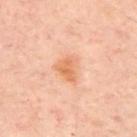Longest diameter approximately 3 mm. Located on the upper back. The subject is in their mid- to late 50s. Cropped from a total-body skin-imaging series; the visible field is about 15 mm. Captured under cross-polarized illumination.